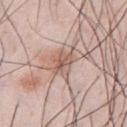Notes:
- workup — total-body-photography surveillance lesion; no biopsy
- lesion size — ≈3.5 mm
- subject — male, in their mid-30s
- body site — the chest
- illumination — white-light illumination
- acquisition — total-body-photography crop, ~15 mm field of view